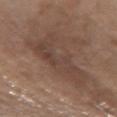Recorded during total-body skin imaging; not selected for excision or biopsy. Measured at roughly 7 mm in maximum diameter. An algorithmic analysis of the crop reported a border-irregularity rating of about 5/10 and internal color variation of about 4 on a 0–10 scale. It also reported a nevus-likeness score of about 0/100 and a detector confidence of about 95 out of 100 that the crop contains a lesion. The lesion is located on the left forearm. The tile uses white-light illumination. A roughly 15 mm field-of-view crop from a total-body skin photograph. A male subject in their mid-60s.